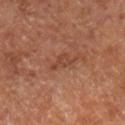No biopsy was performed on this lesion — it was imaged during a full skin examination and was not determined to be concerning.
Automated tile analysis of the lesion measured radial color variation of about 1. The software also gave a nevus-likeness score of about 0/100 and a lesion-detection confidence of about 100/100.
This is a cross-polarized tile.
This image is a 15 mm lesion crop taken from a total-body photograph.
The subject is roughly 65 years of age.
Located on the right lower leg.